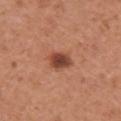  biopsy_status: not biopsied; imaged during a skin examination
  lighting: white-light
  site: right upper arm
  lesion_size:
    long_diameter_mm_approx: 3.0
  patient:
    sex: female
    age_approx: 55
  image:
    source: total-body photography crop
    field_of_view_mm: 15